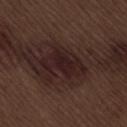{"biopsy_status": "not biopsied; imaged during a skin examination", "lighting": "white-light", "image": {"source": "total-body photography crop", "field_of_view_mm": 15}, "patient": {"sex": "male", "age_approx": 70}, "site": "lower back", "lesion_size": {"long_diameter_mm_approx": 5.5}}